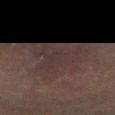- biopsy status — no biopsy performed (imaged during a skin exam)
- acquisition — ~15 mm crop, total-body skin-cancer survey
- lesion size — ~7 mm (longest diameter)
- lighting — cross-polarized
- patient — male, aged around 50
- body site — the abdomen